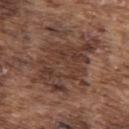workup: catalogued during a skin exam; not biopsied | image: ~15 mm crop, total-body skin-cancer survey | lesion diameter: about 8.5 mm | site: the upper back | patient: male, aged 73 to 77.